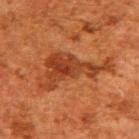notes = total-body-photography surveillance lesion; no biopsy | image = ~15 mm tile from a whole-body skin photo | anatomic site = the upper back | patient = male, in their 60s.